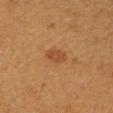  biopsy_status: not biopsied; imaged during a skin examination
  image:
    source: total-body photography crop
    field_of_view_mm: 15
  site: arm
  lighting: cross-polarized
  lesion_size:
    long_diameter_mm_approx: 2.5
  patient:
    sex: female
    age_approx: 40